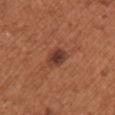Clinical impression:
This lesion was catalogued during total-body skin photography and was not selected for biopsy.
Context:
Approximately 3 mm at its widest. A female patient, roughly 65 years of age. A 15 mm close-up extracted from a 3D total-body photography capture. The lesion-visualizer software estimated an area of roughly 5 mm², an outline eccentricity of about 0.75 (0 = round, 1 = elongated), and a shape-asymmetry score of about 0.25 (0 = symmetric). The analysis additionally found an average lesion color of about L≈38 a*≈24 b*≈28 (CIELAB), a lesion–skin lightness drop of about 11, and a normalized lesion–skin contrast near 10. It also reported a within-lesion color-variation index near 3.5/10 and radial color variation of about 1. The analysis additionally found an automated nevus-likeness rating near 90 out of 100. On the front of the torso.